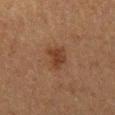Impression: No biopsy was performed on this lesion — it was imaged during a full skin examination and was not determined to be concerning. Context: Automated image analysis of the tile measured an area of roughly 5 mm², an outline eccentricity of about 0.7 (0 = round, 1 = elongated), and two-axis asymmetry of about 0.35. Longest diameter approximately 3 mm. A male subject, in their mid- to late 70s. From the right thigh. A 15 mm close-up tile from a total-body photography series done for melanoma screening.